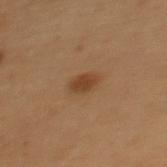Captured during whole-body skin photography for melanoma surveillance; the lesion was not biopsied. A female patient, approximately 50 years of age. The total-body-photography lesion software estimated a mean CIELAB color near L≈35 a*≈18 b*≈30 and a normalized lesion–skin contrast near 8. And it measured a border-irregularity index near 2.5/10. From the back. A region of skin cropped from a whole-body photographic capture, roughly 15 mm wide. Imaged with cross-polarized lighting.